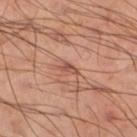location: the leg | lesion diameter: ~2.5 mm (longest diameter) | image: total-body-photography crop, ~15 mm field of view | patient: male, aged approximately 50.